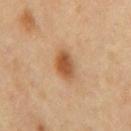Impression:
Part of a total-body skin-imaging series; this lesion was reviewed on a skin check and was not flagged for biopsy.
Acquisition and patient details:
On the mid back. This image is a 15 mm lesion crop taken from a total-body photograph. The subject is a male aged 53 to 57.